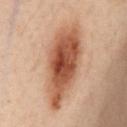This lesion was catalogued during total-body skin photography and was not selected for biopsy.
This image is a 15 mm lesion crop taken from a total-body photograph.
The lesion is located on the abdomen.
Captured under cross-polarized illumination.
About 9.5 mm across.
A female patient aged around 55.
The lesion-visualizer software estimated a lesion area of about 32 mm², an eccentricity of roughly 0.85, and a symmetry-axis asymmetry near 0.2. And it measured a mean CIELAB color near L≈44 a*≈20 b*≈28, about 13 CIELAB-L* units darker than the surrounding skin, and a normalized border contrast of about 10. And it measured a nevus-likeness score of about 95/100.